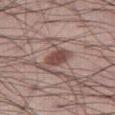biopsy_status: not biopsied; imaged during a skin examination
site: right thigh
automated_metrics:
  area_mm2_approx: 5.5
  eccentricity: 0.8
  shape_asymmetry: 0.15
  nevus_likeness_0_100: 90
  lesion_detection_confidence_0_100: 100
lighting: white-light
image:
  source: total-body photography crop
  field_of_view_mm: 15
patient:
  sex: male
  age_approx: 55